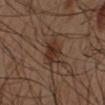Part of a total-body skin-imaging series; this lesion was reviewed on a skin check and was not flagged for biopsy. Cropped from a total-body skin-imaging series; the visible field is about 15 mm. An algorithmic analysis of the crop reported an area of roughly 6.5 mm², a shape eccentricity near 0.65, and a symmetry-axis asymmetry near 0.15. The software also gave border irregularity of about 2 on a 0–10 scale, a within-lesion color-variation index near 3.5/10, and a peripheral color-asymmetry measure near 1.5. Approximately 3.5 mm at its widest. From the arm. Imaged with cross-polarized lighting. A male patient about 50 years old.